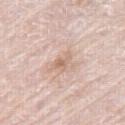Clinical impression:
Recorded during total-body skin imaging; not selected for excision or biopsy.
Image and clinical context:
Automated image analysis of the tile measured a nevus-likeness score of about 0/100 and a lesion-detection confidence of about 100/100. A 15 mm close-up tile from a total-body photography series done for melanoma screening. The lesion is located on the right thigh. The tile uses white-light illumination. A male subject about 80 years old. Longest diameter approximately 2.5 mm.